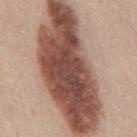The patient is a male in their mid-40s.
From the mid back.
The lesion's longest dimension is about 16.5 mm.
A close-up tile cropped from a whole-body skin photograph, about 15 mm across.
Captured under white-light illumination.